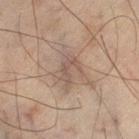Recorded during total-body skin imaging; not selected for excision or biopsy.
The tile uses cross-polarized illumination.
Cropped from a whole-body photographic skin survey; the tile spans about 15 mm.
The patient is a male aged around 45.
The lesion is on the left thigh.
About 3.5 mm across.